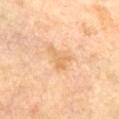biopsy status: catalogued during a skin exam; not biopsied | illumination: cross-polarized | location: the chest | lesion diameter: about 3 mm | image source: ~15 mm tile from a whole-body skin photo | patient: female, roughly 60 years of age.